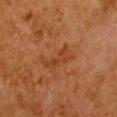No biopsy was performed on this lesion — it was imaged during a full skin examination and was not determined to be concerning.
A female subject, aged around 50.
From the chest.
This is a cross-polarized tile.
Automated tile analysis of the lesion measured an average lesion color of about L≈34 a*≈22 b*≈32 (CIELAB), about 5 CIELAB-L* units darker than the surrounding skin, and a lesion-to-skin contrast of about 5.5 (normalized; higher = more distinct).
Approximately 4 mm at its widest.
This image is a 15 mm lesion crop taken from a total-body photograph.